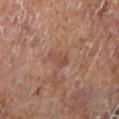location: the left lower leg | lighting: cross-polarized illumination | patient: male, roughly 70 years of age | imaging modality: total-body-photography crop, ~15 mm field of view | TBP lesion metrics: a border-irregularity rating of about 3.5/10 and a within-lesion color-variation index near 1.5/10; an automated nevus-likeness rating near 0 out of 100 and a lesion-detection confidence of about 100/100.